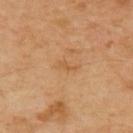Part of a total-body skin-imaging series; this lesion was reviewed on a skin check and was not flagged for biopsy.
Located on the upper back.
About 2.5 mm across.
A 15 mm crop from a total-body photograph taken for skin-cancer surveillance.
A male patient, aged around 50.
Imaged with cross-polarized lighting.